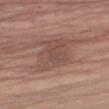biopsy_status: not biopsied; imaged during a skin examination
site: left upper arm
automated_metrics:
  area_mm2_approx: 18.0
  eccentricity: 0.6
  shape_asymmetry: 0.25
  cielab_L: 49
  cielab_a: 19
  cielab_b: 24
  vs_skin_darker_L: 8.0
  vs_skin_contrast_norm: 6.0
  border_irregularity_0_10: 3.5
  color_variation_0_10: 3.0
  peripheral_color_asymmetry: 1.0
image:
  source: total-body photography crop
  field_of_view_mm: 15
patient:
  sex: male
  age_approx: 65